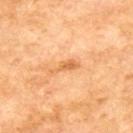| feature | finding |
|---|---|
| biopsy status | total-body-photography surveillance lesion; no biopsy |
| lesion size | ~3.5 mm (longest diameter) |
| image source | total-body-photography crop, ~15 mm field of view |
| subject | male, roughly 70 years of age |
| anatomic site | the upper back |